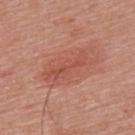Notes:
• notes · imaged on a skin check; not biopsied
• lesion diameter · about 6.5 mm
• TBP lesion metrics · about 7 CIELAB-L* units darker than the surrounding skin and a normalized lesion–skin contrast near 5.5; border irregularity of about 5.5 on a 0–10 scale, a color-variation rating of about 4/10, and peripheral color asymmetry of about 1.5; a nevus-likeness score of about 35/100 and lesion-presence confidence of about 100/100
• site · the upper back
• tile lighting · white-light
• acquisition · ~15 mm crop, total-body skin-cancer survey
• patient · male, in their 50s